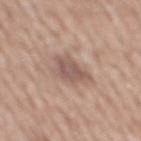No biopsy was performed on this lesion — it was imaged during a full skin examination and was not determined to be concerning. A 15 mm crop from a total-body photograph taken for skin-cancer surveillance. A male patient, aged approximately 65. The lesion is on the mid back.